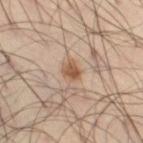The lesion was photographed on a routine skin check and not biopsied; there is no pathology result.
A male patient approximately 45 years of age.
The tile uses cross-polarized illumination.
The lesion is on the right thigh.
About 2.5 mm across.
The lesion-visualizer software estimated a footprint of about 3.5 mm², a shape eccentricity near 0.5, and a symmetry-axis asymmetry near 0.2. And it measured a border-irregularity rating of about 2/10, a color-variation rating of about 3/10, and peripheral color asymmetry of about 1.
This image is a 15 mm lesion crop taken from a total-body photograph.